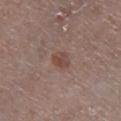Clinical impression: Captured during whole-body skin photography for melanoma surveillance; the lesion was not biopsied. Clinical summary: Automated tile analysis of the lesion measured a footprint of about 4 mm² and two-axis asymmetry of about 0.2. The software also gave a mean CIELAB color near L≈45 a*≈18 b*≈23, about 8 CIELAB-L* units darker than the surrounding skin, and a lesion-to-skin contrast of about 6.5 (normalized; higher = more distinct). Approximately 2.5 mm at its widest. A male subject aged 63–67. The tile uses white-light illumination. Cropped from a total-body skin-imaging series; the visible field is about 15 mm. On the right lower leg.